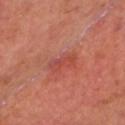Notes:
* workup · imaged on a skin check; not biopsied
* patient · male, aged around 65
* acquisition · total-body-photography crop, ~15 mm field of view
* tile lighting · cross-polarized illumination
* location · the chest
* lesion diameter · ≈3.5 mm